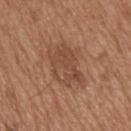notes: total-body-photography surveillance lesion; no biopsy | imaging modality: total-body-photography crop, ~15 mm field of view | illumination: white-light | anatomic site: the upper back | subject: male, approximately 65 years of age.